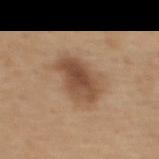This lesion was catalogued during total-body skin photography and was not selected for biopsy. On the back. A female subject approximately 45 years of age. This is a white-light tile. Longest diameter approximately 5 mm. A lesion tile, about 15 mm wide, cut from a 3D total-body photograph.